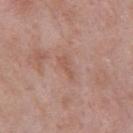This lesion was catalogued during total-body skin photography and was not selected for biopsy. A roughly 15 mm field-of-view crop from a total-body skin photograph. The total-body-photography lesion software estimated a border-irregularity index near 5/10, a within-lesion color-variation index near 0/10, and peripheral color asymmetry of about 0. The software also gave lesion-presence confidence of about 100/100. Measured at roughly 3 mm in maximum diameter. Captured under white-light illumination. Located on the chest. A male patient aged around 55.